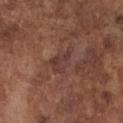notes=no biopsy performed (imaged during a skin exam); location=the chest; patient=male, aged 73 to 77; diameter=≈3.5 mm; acquisition=~15 mm crop, total-body skin-cancer survey; tile lighting=white-light illumination.